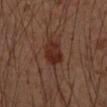The lesion was tiled from a total-body skin photograph and was not biopsied. Measured at roughly 3.5 mm in maximum diameter. On the right upper arm. Cropped from a total-body skin-imaging series; the visible field is about 15 mm. A male patient aged 48 to 52. Automated image analysis of the tile measured a lesion area of about 7 mm², an outline eccentricity of about 0.65 (0 = round, 1 = elongated), and two-axis asymmetry of about 0.2. The analysis additionally found a border-irregularity rating of about 2/10 and radial color variation of about 1. It also reported an automated nevus-likeness rating near 70 out of 100 and lesion-presence confidence of about 100/100. Imaged with cross-polarized lighting.